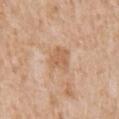biopsy_status: not biopsied; imaged during a skin examination
site: chest
lesion_size:
  long_diameter_mm_approx: 3.0
patient:
  sex: male
  age_approx: 65
lighting: white-light
image:
  source: total-body photography crop
  field_of_view_mm: 15